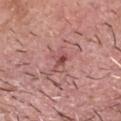No biopsy was performed on this lesion — it was imaged during a full skin examination and was not determined to be concerning.
Measured at roughly 2.5 mm in maximum diameter.
A male patient aged approximately 50.
The lesion is located on the head or neck.
Imaged with white-light lighting.
Automated tile analysis of the lesion measured an eccentricity of roughly 0.75 and two-axis asymmetry of about 0.4. And it measured a lesion color around L≈50 a*≈27 b*≈22 in CIELAB and about 9 CIELAB-L* units darker than the surrounding skin. The software also gave a border-irregularity index near 4/10 and peripheral color asymmetry of about 1.
A region of skin cropped from a whole-body photographic capture, roughly 15 mm wide.